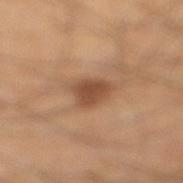- tile lighting — cross-polarized
- subject — male, aged 38 to 42
- imaging modality — 15 mm crop, total-body photography
- size — ~3.5 mm (longest diameter)
- site — the leg